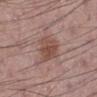Clinical impression:
Captured during whole-body skin photography for melanoma surveillance; the lesion was not biopsied.